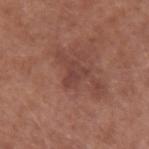Q: How was the tile lit?
A: white-light
Q: Where on the body is the lesion?
A: the right forearm
Q: Patient demographics?
A: male, aged approximately 60
Q: What is the lesion's diameter?
A: ~3 mm (longest diameter)
Q: What kind of image is this?
A: ~15 mm crop, total-body skin-cancer survey